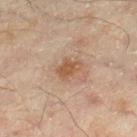No biopsy was performed on this lesion — it was imaged during a full skin examination and was not determined to be concerning.
A male patient in their mid-40s.
A close-up tile cropped from a whole-body skin photograph, about 15 mm across.
The recorded lesion diameter is about 3 mm.
Located on the right lower leg.
This is a cross-polarized tile.